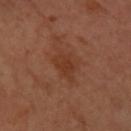Clinical impression: The lesion was photographed on a routine skin check and not biopsied; there is no pathology result. Background: The total-body-photography lesion software estimated a lesion area of about 6.5 mm², an outline eccentricity of about 0.7 (0 = round, 1 = elongated), and a symmetry-axis asymmetry near 0.35. The software also gave a classifier nevus-likeness of about 0/100. A lesion tile, about 15 mm wide, cut from a 3D total-body photograph. The subject is a female about 50 years old. The recorded lesion diameter is about 3.5 mm. Captured under cross-polarized illumination. Located on the arm.